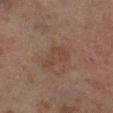No biopsy was performed on this lesion — it was imaged during a full skin examination and was not determined to be concerning. A male subject, aged 68 to 72. Longest diameter approximately 4 mm. Captured under cross-polarized illumination. Automated image analysis of the tile measured an automated nevus-likeness rating near 0 out of 100 and a lesion-detection confidence of about 100/100. Located on the left lower leg. This image is a 15 mm lesion crop taken from a total-body photograph.